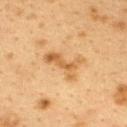{
  "biopsy_status": "not biopsied; imaged during a skin examination",
  "image": {
    "source": "total-body photography crop",
    "field_of_view_mm": 15
  },
  "patient": {
    "sex": "female",
    "age_approx": 40
  },
  "lesion_size": {
    "long_diameter_mm_approx": 4.5
  },
  "site": "upper back",
  "lighting": "cross-polarized"
}